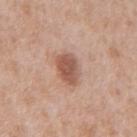Q: Automated lesion metrics?
A: a classifier nevus-likeness of about 95/100 and a detector confidence of about 100 out of 100 that the crop contains a lesion
Q: How large is the lesion?
A: ~3.5 mm (longest diameter)
Q: Where on the body is the lesion?
A: the mid back
Q: What kind of image is this?
A: ~15 mm tile from a whole-body skin photo
Q: Illumination type?
A: white-light illumination
Q: Patient demographics?
A: male, in their mid-60s